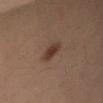Q: Is there a histopathology result?
A: no biopsy performed (imaged during a skin exam)
Q: What are the patient's age and sex?
A: male, aged approximately 35
Q: How was the tile lit?
A: cross-polarized
Q: What kind of image is this?
A: ~15 mm tile from a whole-body skin photo
Q: Where on the body is the lesion?
A: the right upper arm